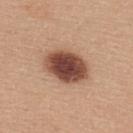* follow-up — imaged on a skin check; not biopsied
* tile lighting — white-light illumination
* imaging modality — total-body-photography crop, ~15 mm field of view
* patient — female, aged approximately 30
* size — ~5.5 mm (longest diameter)
* site — the upper back
* TBP lesion metrics — an outline eccentricity of about 0.7 (0 = round, 1 = elongated) and two-axis asymmetry of about 0.1; a mean CIELAB color near L≈47 a*≈22 b*≈28, about 19 CIELAB-L* units darker than the surrounding skin, and a normalized border contrast of about 13; internal color variation of about 5.5 on a 0–10 scale and peripheral color asymmetry of about 1.5; an automated nevus-likeness rating near 95 out of 100 and lesion-presence confidence of about 100/100